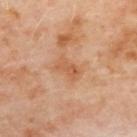Background:
A roughly 15 mm field-of-view crop from a total-body skin photograph. An algorithmic analysis of the crop reported a lesion color around L≈58 a*≈24 b*≈37 in CIELAB, a lesion–skin lightness drop of about 8, and a normalized lesion–skin contrast near 6. The lesion is on the upper back. About 3 mm across. A female subject, aged 58–62.